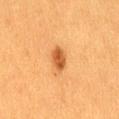The lesion was tiled from a total-body skin photograph and was not biopsied.
A female patient roughly 40 years of age.
A 15 mm close-up tile from a total-body photography series done for melanoma screening.
On the back.
About 2.5 mm across.
Captured under cross-polarized illumination.